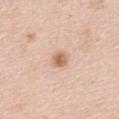Part of a total-body skin-imaging series; this lesion was reviewed on a skin check and was not flagged for biopsy.
Located on the back.
The patient is a female about 65 years old.
A 15 mm close-up tile from a total-body photography series done for melanoma screening.